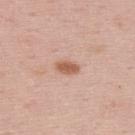The lesion was photographed on a routine skin check and not biopsied; there is no pathology result.
Automated tile analysis of the lesion measured a lesion area of about 4 mm² and an eccentricity of roughly 0.8. And it measured a border-irregularity rating of about 1.5/10, a color-variation rating of about 1.5/10, and a peripheral color-asymmetry measure near 0.5. The software also gave a classifier nevus-likeness of about 100/100 and a lesion-detection confidence of about 100/100.
The lesion is located on the upper back.
A male patient, aged approximately 50.
A roughly 15 mm field-of-view crop from a total-body skin photograph.
Captured under white-light illumination.